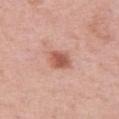Case summary:
* notes — catalogued during a skin exam; not biopsied
* anatomic site — the left thigh
* patient — female, approximately 40 years of age
* diameter — ~3 mm (longest diameter)
* image source — total-body-photography crop, ~15 mm field of view